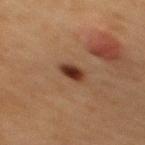Q: Was a biopsy performed?
A: catalogued during a skin exam; not biopsied
Q: What kind of image is this?
A: ~15 mm tile from a whole-body skin photo
Q: Illumination type?
A: cross-polarized illumination
Q: What is the lesion's diameter?
A: ~3 mm (longest diameter)
Q: Where on the body is the lesion?
A: the mid back
Q: Who is the patient?
A: female, aged 38–42